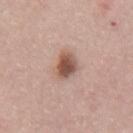Impression: No biopsy was performed on this lesion — it was imaged during a full skin examination and was not determined to be concerning. Background: Cropped from a whole-body photographic skin survey; the tile spans about 15 mm. Located on the mid back. The subject is a male approximately 65 years of age.